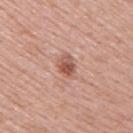No biopsy was performed on this lesion — it was imaged during a full skin examination and was not determined to be concerning. Located on the upper back. A male patient, about 55 years old. Automated image analysis of the tile measured a mean CIELAB color near L≈55 a*≈24 b*≈29, roughly 12 lightness units darker than nearby skin, and a normalized lesion–skin contrast near 8. The software also gave border irregularity of about 3 on a 0–10 scale and a color-variation rating of about 5.5/10. It also reported an automated nevus-likeness rating near 85 out of 100 and a lesion-detection confidence of about 100/100. About 3 mm across. This is a white-light tile. Cropped from a total-body skin-imaging series; the visible field is about 15 mm.